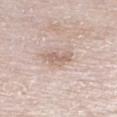notes: total-body-photography surveillance lesion; no biopsy
patient: male, aged approximately 80
illumination: white-light
anatomic site: the right lower leg
imaging modality: ~15 mm crop, total-body skin-cancer survey